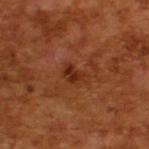{"biopsy_status": "not biopsied; imaged during a skin examination", "patient": {"sex": "male", "age_approx": 65}, "lesion_size": {"long_diameter_mm_approx": 3.0}, "image": {"source": "total-body photography crop", "field_of_view_mm": 15}, "lighting": "cross-polarized"}